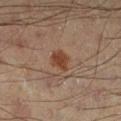Imaged during a routine full-body skin examination; the lesion was not biopsied and no histopathology is available. A male patient, roughly 40 years of age. About 2.5 mm across. The lesion is located on the left lower leg. A 15 mm close-up extracted from a 3D total-body photography capture. Automated tile analysis of the lesion measured a lesion color around L≈37 a*≈19 b*≈27 in CIELAB, roughly 9 lightness units darker than nearby skin, and a normalized lesion–skin contrast near 8.5. The software also gave a border-irregularity index near 2/10, a within-lesion color-variation index near 1.5/10, and peripheral color asymmetry of about 0.5.